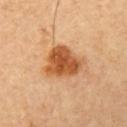Imaged during a routine full-body skin examination; the lesion was not biopsied and no histopathology is available. Automated image analysis of the tile measured a classifier nevus-likeness of about 100/100 and a detector confidence of about 100 out of 100 that the crop contains a lesion. Approximately 4.5 mm at its widest. The patient is a male roughly 65 years of age. The lesion is on the arm. A lesion tile, about 15 mm wide, cut from a 3D total-body photograph.